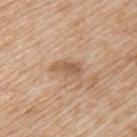follow-up = total-body-photography surveillance lesion; no biopsy | anatomic site = the back | subject = male, aged around 65 | acquisition = ~15 mm crop, total-body skin-cancer survey | tile lighting = white-light.